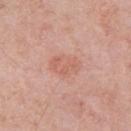| key | value |
|---|---|
| biopsy status | total-body-photography surveillance lesion; no biopsy |
| subject | male, aged approximately 70 |
| acquisition | ~15 mm tile from a whole-body skin photo |
| lesion size | about 3.5 mm |
| anatomic site | the arm |